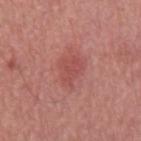Notes:
– notes: no biopsy performed (imaged during a skin exam)
– automated lesion analysis: a lesion–skin lightness drop of about 7 and a normalized lesion–skin contrast near 5; internal color variation of about 2 on a 0–10 scale and radial color variation of about 1
– subject: male, roughly 65 years of age
– anatomic site: the mid back
– acquisition: ~15 mm crop, total-body skin-cancer survey
– illumination: white-light